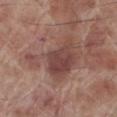Assessment:
Imaged during a routine full-body skin examination; the lesion was not biopsied and no histopathology is available.
Image and clinical context:
From the left lower leg. A male patient about 70 years old. A close-up tile cropped from a whole-body skin photograph, about 15 mm across. Approximately 7.5 mm at its widest.